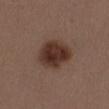The lesion was tiled from a total-body skin photograph and was not biopsied.
On the left thigh.
The tile uses white-light illumination.
A 15 mm crop from a total-body photograph taken for skin-cancer surveillance.
Longest diameter approximately 5 mm.
Automated tile analysis of the lesion measured a symmetry-axis asymmetry near 0.15. It also reported a lesion–skin lightness drop of about 12. It also reported a within-lesion color-variation index near 4.5/10.
The subject is a female aged 38 to 42.